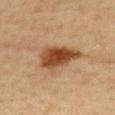patient — female, about 45 years old | image — 15 mm crop, total-body photography | site — the upper back.